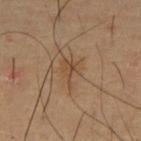Case summary:
• biopsy status: imaged on a skin check; not biopsied
• tile lighting: cross-polarized
• TBP lesion metrics: an area of roughly 6 mm² and a shape eccentricity near 0.7; a lesion color around L≈46 a*≈16 b*≈31 in CIELAB, a lesion–skin lightness drop of about 6, and a lesion-to-skin contrast of about 5.5 (normalized; higher = more distinct); a nevus-likeness score of about 0/100 and a detector confidence of about 100 out of 100 that the crop contains a lesion
• subject: male, about 55 years old
• image: ~15 mm tile from a whole-body skin photo
• site: the right forearm